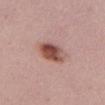| field | value |
|---|---|
| notes | catalogued during a skin exam; not biopsied |
| subject | female, aged around 45 |
| body site | the chest |
| image | ~15 mm tile from a whole-body skin photo |
| image-analysis metrics | a lesion color around L≈51 a*≈23 b*≈25 in CIELAB, roughly 14 lightness units darker than nearby skin, and a lesion-to-skin contrast of about 10 (normalized; higher = more distinct); a nevus-likeness score of about 100/100 |